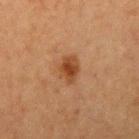Q: Was this lesion biopsied?
A: imaged on a skin check; not biopsied
Q: What lighting was used for the tile?
A: cross-polarized
Q: How was this image acquired?
A: ~15 mm tile from a whole-body skin photo
Q: Where on the body is the lesion?
A: the right upper arm
Q: What are the patient's age and sex?
A: female, aged 38–42
Q: How large is the lesion?
A: about 3 mm